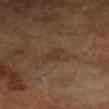biopsy_status: not biopsied; imaged during a skin examination
lesion_size:
  long_diameter_mm_approx: 3.0
lighting: cross-polarized
patient:
  sex: male
  age_approx: 65
image:
  source: total-body photography crop
  field_of_view_mm: 15